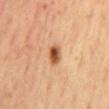Imaged during a routine full-body skin examination; the lesion was not biopsied and no histopathology is available. The lesion is on the mid back. Cropped from a whole-body photographic skin survey; the tile spans about 15 mm. The lesion's longest dimension is about 2.5 mm. The total-body-photography lesion software estimated about 15 CIELAB-L* units darker than the surrounding skin. The analysis additionally found an automated nevus-likeness rating near 100 out of 100 and a lesion-detection confidence of about 100/100. A male subject, approximately 65 years of age. The tile uses cross-polarized illumination.